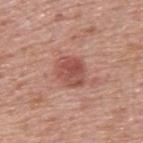Background: Longest diameter approximately 4 mm. A male subject aged around 75. Located on the upper back. Cropped from a total-body skin-imaging series; the visible field is about 15 mm.